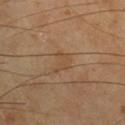<case>
  <biopsy_status>not biopsied; imaged during a skin examination</biopsy_status>
  <image>
    <source>total-body photography crop</source>
    <field_of_view_mm>15</field_of_view_mm>
  </image>
  <lighting>cross-polarized</lighting>
  <automated_metrics>
    <area_mm2_approx>4.0</area_mm2_approx>
    <shape_asymmetry>0.55</shape_asymmetry>
    <vs_skin_darker_L>5.0</vs_skin_darker_L>
    <vs_skin_contrast_norm>4.5</vs_skin_contrast_norm>
    <lesion_detection_confidence_0_100>100</lesion_detection_confidence_0_100>
  </automated_metrics>
  <patient>
    <sex>male</sex>
    <age_approx>65</age_approx>
  </patient>
  <site>leg</site>
</case>